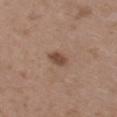Imaged during a routine full-body skin examination; the lesion was not biopsied and no histopathology is available. Located on the upper back. A female subject roughly 35 years of age. A 15 mm crop from a total-body photograph taken for skin-cancer surveillance. Approximately 2.5 mm at its widest. Captured under white-light illumination.